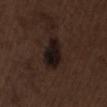Impression: No biopsy was performed on this lesion — it was imaged during a full skin examination and was not determined to be concerning. Context: An algorithmic analysis of the crop reported an eccentricity of roughly 0.9 and a shape-asymmetry score of about 0.2 (0 = symmetric). It also reported about 9 CIELAB-L* units darker than the surrounding skin and a normalized border contrast of about 13.5. About 5.5 mm across. A male patient aged approximately 70. Imaged with white-light lighting. A lesion tile, about 15 mm wide, cut from a 3D total-body photograph. From the lower back.